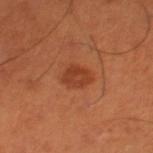Imaged during a routine full-body skin examination; the lesion was not biopsied and no histopathology is available. The patient is a male aged 48–52. The lesion-visualizer software estimated a lesion area of about 6 mm², an outline eccentricity of about 0.65 (0 = round, 1 = elongated), and two-axis asymmetry of about 0.2. It also reported a lesion color around L≈41 a*≈29 b*≈36 in CIELAB, about 8 CIELAB-L* units darker than the surrounding skin, and a normalized lesion–skin contrast near 6.5. The software also gave a border-irregularity rating of about 2/10 and radial color variation of about 1. This image is a 15 mm lesion crop taken from a total-body photograph. This is a cross-polarized tile. Located on the right thigh.